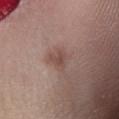{
  "biopsy_status": "not biopsied; imaged during a skin examination",
  "image": {
    "source": "total-body photography crop",
    "field_of_view_mm": 15
  },
  "patient": {
    "sex": "female",
    "age_approx": 40
  },
  "lesion_size": {
    "long_diameter_mm_approx": 2.5
  },
  "lighting": "white-light",
  "site": "leg"
}